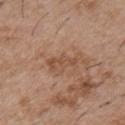The lesion was photographed on a routine skin check and not biopsied; there is no pathology result.